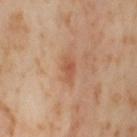Impression: No biopsy was performed on this lesion — it was imaged during a full skin examination and was not determined to be concerning. Acquisition and patient details: From the left thigh. A female patient, in their mid-50s. Automated tile analysis of the lesion measured a lesion color around L≈56 a*≈24 b*≈34 in CIELAB, roughly 8 lightness units darker than nearby skin, and a normalized lesion–skin contrast near 6. It also reported a nevus-likeness score of about 25/100. A 15 mm close-up extracted from a 3D total-body photography capture. Imaged with cross-polarized lighting. Measured at roughly 3 mm in maximum diameter.